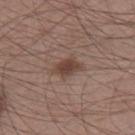A male patient, aged approximately 45. The lesion-visualizer software estimated a lesion area of about 5.5 mm² and two-axis asymmetry of about 0.25. And it measured a border-irregularity rating of about 2.5/10 and internal color variation of about 2.5 on a 0–10 scale. This image is a 15 mm lesion crop taken from a total-body photograph. The lesion's longest dimension is about 3.5 mm. The tile uses white-light illumination. The lesion is on the leg.